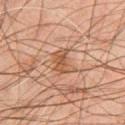| feature | finding |
|---|---|
| workup | imaged on a skin check; not biopsied |
| image | ~15 mm crop, total-body skin-cancer survey |
| site | the chest |
| subject | male, aged 43–47 |